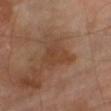{
  "biopsy_status": "not biopsied; imaged during a skin examination",
  "image": {
    "source": "total-body photography crop",
    "field_of_view_mm": 15
  },
  "patient": {
    "sex": "male",
    "age_approx": 70
  },
  "site": "right thigh"
}